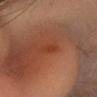| field | value |
|---|---|
| notes | no biopsy performed (imaged during a skin exam) |
| subject | female, about 35 years old |
| site | the head or neck |
| tile lighting | cross-polarized illumination |
| size | ~2.5 mm (longest diameter) |
| imaging modality | ~15 mm tile from a whole-body skin photo |